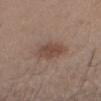Captured during whole-body skin photography for melanoma surveillance; the lesion was not biopsied. The lesion's longest dimension is about 3.5 mm. This is a white-light tile. Cropped from a total-body skin-imaging series; the visible field is about 15 mm. The lesion is located on the head or neck. The subject is a male in their 30s. The total-body-photography lesion software estimated an area of roughly 8 mm², an eccentricity of roughly 0.65, and two-axis asymmetry of about 0.2. The software also gave a lesion color around L≈46 a*≈17 b*≈24 in CIELAB, a lesion–skin lightness drop of about 9, and a lesion-to-skin contrast of about 7 (normalized; higher = more distinct). The software also gave a border-irregularity rating of about 2.5/10 and radial color variation of about 1. The software also gave a nevus-likeness score of about 55/100.